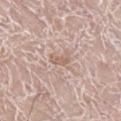Captured during whole-body skin photography for melanoma surveillance; the lesion was not biopsied.
The lesion is on the left leg.
Longest diameter approximately 2.5 mm.
Cropped from a total-body skin-imaging series; the visible field is about 15 mm.
The subject is a male aged 78 to 82.